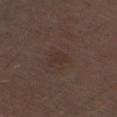This is a white-light tile.
The lesion is located on the right thigh.
A male subject, aged 68–72.
A 15 mm close-up extracted from a 3D total-body photography capture.
About 2.5 mm across.